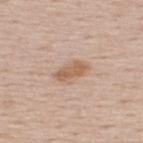Assessment:
Captured during whole-body skin photography for melanoma surveillance; the lesion was not biopsied.
Acquisition and patient details:
A male patient roughly 60 years of age. A close-up tile cropped from a whole-body skin photograph, about 15 mm across. The lesion's longest dimension is about 4 mm. On the upper back. Captured under white-light illumination.